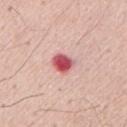Clinical impression: This lesion was catalogued during total-body skin photography and was not selected for biopsy. Clinical summary: A male subject aged approximately 55. A roughly 15 mm field-of-view crop from a total-body skin photograph.